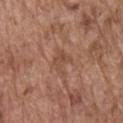Impression: Captured during whole-body skin photography for melanoma surveillance; the lesion was not biopsied. Acquisition and patient details: A male patient roughly 75 years of age. Cropped from a whole-body photographic skin survey; the tile spans about 15 mm. Longest diameter approximately 3 mm. The lesion is located on the chest. This is a white-light tile.